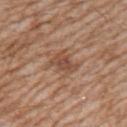Findings:
* workup: imaged on a skin check; not biopsied
* patient: male, roughly 60 years of age
* site: the right upper arm
* image source: total-body-photography crop, ~15 mm field of view